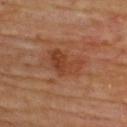Clinical impression:
Captured during whole-body skin photography for melanoma surveillance; the lesion was not biopsied.
Image and clinical context:
The patient is a male approximately 60 years of age. A region of skin cropped from a whole-body photographic capture, roughly 15 mm wide. Located on the back.